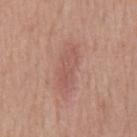| key | value |
|---|---|
| follow-up | imaged on a skin check; not biopsied |